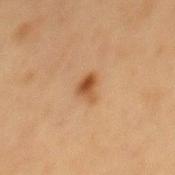follow-up: imaged on a skin check; not biopsied
image source: ~15 mm tile from a whole-body skin photo
automated metrics: a shape-asymmetry score of about 0.4 (0 = symmetric); a mean CIELAB color near L≈44 a*≈20 b*≈34 and roughly 11 lightness units darker than nearby skin
location: the mid back
size: ~2.5 mm (longest diameter)
subject: female, aged around 40
tile lighting: cross-polarized